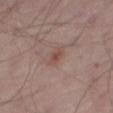workup=imaged on a skin check; not biopsied
subject=male, in their mid-60s
automated lesion analysis=a footprint of about 3 mm², an eccentricity of roughly 0.9, and a symmetry-axis asymmetry near 0.35; border irregularity of about 3 on a 0–10 scale and a within-lesion color-variation index near 1.5/10
tile lighting=white-light illumination
size=about 3 mm
body site=the leg
acquisition=~15 mm tile from a whole-body skin photo